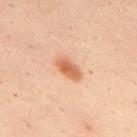| feature | finding |
|---|---|
| biopsy status | catalogued during a skin exam; not biopsied |
| image-analysis metrics | an area of roughly 5 mm²; a lesion–skin lightness drop of about 12 and a lesion-to-skin contrast of about 8.5 (normalized; higher = more distinct) |
| acquisition | ~15 mm tile from a whole-body skin photo |
| body site | the upper back |
| subject | male, approximately 50 years of age |
| tile lighting | cross-polarized illumination |